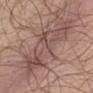follow-up: no biopsy performed (imaged during a skin exam)
lesion size: about 8.5 mm
image source: ~15 mm crop, total-body skin-cancer survey
image-analysis metrics: a footprint of about 25 mm², an outline eccentricity of about 0.9 (0 = round, 1 = elongated), and a shape-asymmetry score of about 0.5 (0 = symmetric); a lesion color around L≈49 a*≈19 b*≈22 in CIELAB and a normalized border contrast of about 6; internal color variation of about 6 on a 0–10 scale and peripheral color asymmetry of about 2.5
site: the chest
subject: male, aged 63 to 67
lighting: white-light illumination